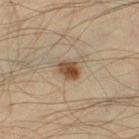Q: Is there a histopathology result?
A: imaged on a skin check; not biopsied
Q: What is the lesion's diameter?
A: about 2.5 mm
Q: What is the anatomic site?
A: the right thigh
Q: How was the tile lit?
A: cross-polarized illumination
Q: How was this image acquired?
A: 15 mm crop, total-body photography
Q: Patient demographics?
A: male, in their mid- to late 30s